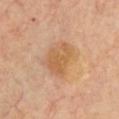Clinical impression:
Recorded during total-body skin imaging; not selected for excision or biopsy.